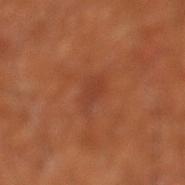biopsy_status: not biopsied; imaged during a skin examination
automated_metrics:
  eccentricity: 0.7
  shape_asymmetry: 0.3
  border_irregularity_0_10: 3.5
  color_variation_0_10: 2.0
  peripheral_color_asymmetry: 0.5
  nevus_likeness_0_100: 0
patient:
  age_approx: 65
lighting: cross-polarized
image:
  source: total-body photography crop
  field_of_view_mm: 15
site: left lower leg
lesion_size:
  long_diameter_mm_approx: 3.0